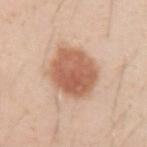{"biopsy_status": "not biopsied; imaged during a skin examination", "lighting": "white-light", "site": "left upper arm", "automated_metrics": {"cielab_L": 60, "cielab_a": 22, "cielab_b": 32, "vs_skin_darker_L": 14.0, "vs_skin_contrast_norm": 9.0, "border_irregularity_0_10": 1.5, "color_variation_0_10": 4.5, "peripheral_color_asymmetry": 1.5}, "image": {"source": "total-body photography crop", "field_of_view_mm": 15}, "patient": {"sex": "male", "age_approx": 30}, "lesion_size": {"long_diameter_mm_approx": 5.5}}